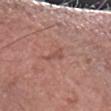Imaged with white-light lighting. A 15 mm close-up extracted from a 3D total-body photography capture. A male patient aged 58–62. Measured at roughly 2.5 mm in maximum diameter. From the left forearm.